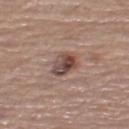Q: Was this lesion biopsied?
A: no biopsy performed (imaged during a skin exam)
Q: What is the anatomic site?
A: the left thigh
Q: What are the patient's age and sex?
A: female, aged around 65
Q: What is the imaging modality?
A: ~15 mm tile from a whole-body skin photo
Q: Lesion size?
A: ~3.5 mm (longest diameter)